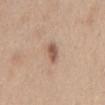{
  "biopsy_status": "not biopsied; imaged during a skin examination",
  "patient": {
    "sex": "female",
    "age_approx": 40
  },
  "lighting": "white-light",
  "site": "abdomen",
  "image": {
    "source": "total-body photography crop",
    "field_of_view_mm": 15
  }
}